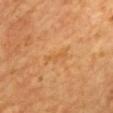{"biopsy_status": "not biopsied; imaged during a skin examination", "lesion_size": {"long_diameter_mm_approx": 3.0}, "lighting": "cross-polarized", "patient": {"sex": "male", "age_approx": 65}, "image": {"source": "total-body photography crop", "field_of_view_mm": 15}, "site": "mid back"}